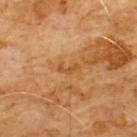Notes:
- workup: imaged on a skin check; not biopsied
- patient: male, in their 60s
- illumination: cross-polarized
- automated lesion analysis: a symmetry-axis asymmetry near 0.65; about 7 CIELAB-L* units darker than the surrounding skin and a normalized border contrast of about 6; a border-irregularity rating of about 6.5/10 and a within-lesion color-variation index near 0/10; an automated nevus-likeness rating near 0 out of 100 and a lesion-detection confidence of about 100/100
- diameter: ≈2.5 mm
- body site: the chest
- image source: 15 mm crop, total-body photography